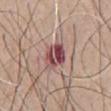notes: total-body-photography surveillance lesion; no biopsy | TBP lesion metrics: a shape eccentricity near 0.8 and two-axis asymmetry of about 0.2 | lighting: white-light | site: the chest | subject: male, in their mid-60s | size: ~4 mm (longest diameter) | image source: total-body-photography crop, ~15 mm field of view.